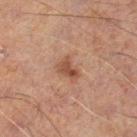Recorded during total-body skin imaging; not selected for excision or biopsy.
A 15 mm crop from a total-body photograph taken for skin-cancer surveillance.
A male subject, aged 58–62.
From the leg.
The recorded lesion diameter is about 2.5 mm.
The total-body-photography lesion software estimated a lesion area of about 4 mm² and a symmetry-axis asymmetry near 0.45. It also reported a lesion–skin lightness drop of about 8 and a lesion-to-skin contrast of about 8 (normalized; higher = more distinct). And it measured a border-irregularity index near 4/10 and a peripheral color-asymmetry measure near 0.5. And it measured a nevus-likeness score of about 15/100 and a lesion-detection confidence of about 100/100.
The tile uses cross-polarized illumination.